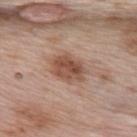follow-up=imaged on a skin check; not biopsied | lighting=white-light illumination | imaging modality=~15 mm crop, total-body skin-cancer survey | lesion size=≈4 mm | subject=female, roughly 65 years of age | location=the upper back.